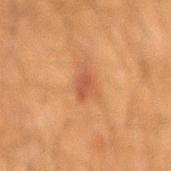Q: Was this lesion biopsied?
A: no biopsy performed (imaged during a skin exam)
Q: What did automated image analysis measure?
A: a lesion color around L≈42 a*≈22 b*≈30 in CIELAB, a lesion–skin lightness drop of about 7, and a lesion-to-skin contrast of about 6.5 (normalized; higher = more distinct)
Q: Who is the patient?
A: male, roughly 60 years of age
Q: What is the lesion's diameter?
A: ≈3 mm
Q: Illumination type?
A: cross-polarized
Q: How was this image acquired?
A: 15 mm crop, total-body photography
Q: Where on the body is the lesion?
A: the mid back